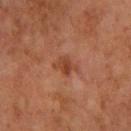  site: left forearm
  patient:
    sex: female
    age_approx: 60
  image:
    source: total-body photography crop
    field_of_view_mm: 15